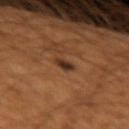Q: What is the imaging modality?
A: ~15 mm crop, total-body skin-cancer survey
Q: Automated lesion metrics?
A: a lesion area of about 3 mm², an eccentricity of roughly 0.75, and two-axis asymmetry of about 0.2; an average lesion color of about L≈31 a*≈19 b*≈27 (CIELAB), about 11 CIELAB-L* units darker than the surrounding skin, and a normalized lesion–skin contrast near 10.5
Q: Illumination type?
A: cross-polarized illumination
Q: Patient demographics?
A: male, aged 48–52
Q: What is the anatomic site?
A: the left forearm